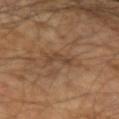Q: Is there a histopathology result?
A: catalogued during a skin exam; not biopsied
Q: What kind of image is this?
A: ~15 mm crop, total-body skin-cancer survey
Q: What did automated image analysis measure?
A: a lesion area of about 3.5 mm² and a shape-asymmetry score of about 0.55 (0 = symmetric); an average lesion color of about L≈40 a*≈16 b*≈28 (CIELAB) and a normalized border contrast of about 6
Q: What are the patient's age and sex?
A: male, in their mid-60s
Q: What is the lesion's diameter?
A: ≈3 mm
Q: Illumination type?
A: cross-polarized
Q: Where on the body is the lesion?
A: the arm